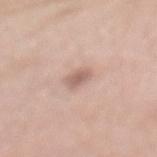workup: imaged on a skin check; not biopsied | subject: female, aged 68 to 72 | lesion size: ~2.5 mm (longest diameter) | image: ~15 mm tile from a whole-body skin photo | site: the back | tile lighting: white-light illumination.